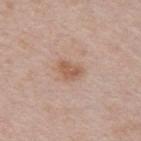Impression:
The lesion was photographed on a routine skin check and not biopsied; there is no pathology result.
Background:
Cropped from a total-body skin-imaging series; the visible field is about 15 mm. The patient is a female approximately 65 years of age. The tile uses white-light illumination. The lesion is located on the upper back. Longest diameter approximately 3 mm.